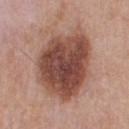{
  "biopsy_status": "not biopsied; imaged during a skin examination",
  "site": "upper back",
  "automated_metrics": {
    "area_mm2_approx": 42.0,
    "shape_asymmetry": 0.15,
    "vs_skin_darker_L": 16.0,
    "vs_skin_contrast_norm": 11.0,
    "border_irregularity_0_10": 2.0,
    "peripheral_color_asymmetry": 2.0,
    "nevus_likeness_0_100": 15,
    "lesion_detection_confidence_0_100": 100
  },
  "lighting": "white-light",
  "lesion_size": {
    "long_diameter_mm_approx": 8.5
  },
  "image": {
    "source": "total-body photography crop",
    "field_of_view_mm": 15
  },
  "patient": {
    "sex": "male",
    "age_approx": 55
  }
}